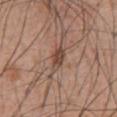<record>
  <biopsy_status>not biopsied; imaged during a skin examination</biopsy_status>
  <lesion_size>
    <long_diameter_mm_approx>3.0</long_diameter_mm_approx>
  </lesion_size>
  <image>
    <source>total-body photography crop</source>
    <field_of_view_mm>15</field_of_view_mm>
  </image>
  <site>abdomen</site>
  <patient>
    <sex>male</sex>
    <age_approx>55</age_approx>
  </patient>
</record>